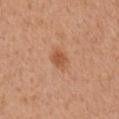Recorded during total-body skin imaging; not selected for excision or biopsy.